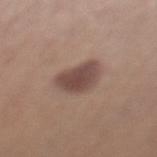Q: Was this lesion biopsied?
A: no biopsy performed (imaged during a skin exam)
Q: Lesion size?
A: ~4 mm (longest diameter)
Q: What are the patient's age and sex?
A: female, roughly 55 years of age
Q: Lesion location?
A: the left lower leg
Q: How was this image acquired?
A: total-body-photography crop, ~15 mm field of view
Q: Illumination type?
A: white-light illumination